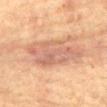Imaged during a routine full-body skin examination; the lesion was not biopsied and no histopathology is available. Imaged with cross-polarized lighting. Measured at roughly 8.5 mm in maximum diameter. A 15 mm crop from a total-body photograph taken for skin-cancer surveillance. A male patient, roughly 65 years of age. The lesion is located on the front of the torso. The total-body-photography lesion software estimated a border-irregularity rating of about 4/10, a within-lesion color-variation index near 4.5/10, and a peripheral color-asymmetry measure near 1.5. It also reported lesion-presence confidence of about 100/100.